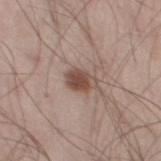workup — total-body-photography surveillance lesion; no biopsy
tile lighting — white-light
site — the left thigh
lesion diameter — ≈3 mm
image-analysis metrics — a footprint of about 5.5 mm², an eccentricity of roughly 0.7, and a shape-asymmetry score of about 0.25 (0 = symmetric); an average lesion color of about L≈48 a*≈18 b*≈24 (CIELAB), about 12 CIELAB-L* units darker than the surrounding skin, and a lesion-to-skin contrast of about 9 (normalized; higher = more distinct); border irregularity of about 2 on a 0–10 scale, a color-variation rating of about 4/10, and peripheral color asymmetry of about 1.5
imaging modality — 15 mm crop, total-body photography
patient — male, aged 28 to 32